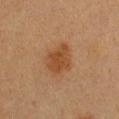The lesion was photographed on a routine skin check and not biopsied; there is no pathology result. The subject is a female aged 38–42. Automated image analysis of the tile measured two-axis asymmetry of about 0.2. It also reported a border-irregularity rating of about 2/10 and a within-lesion color-variation index near 2.5/10. The analysis additionally found a classifier nevus-likeness of about 85/100 and a lesion-detection confidence of about 100/100. About 4 mm across. The lesion is on the chest. A 15 mm close-up tile from a total-body photography series done for melanoma screening. Imaged with cross-polarized lighting.